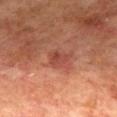Assessment: Part of a total-body skin-imaging series; this lesion was reviewed on a skin check and was not flagged for biopsy. Acquisition and patient details: Cropped from a total-body skin-imaging series; the visible field is about 15 mm. The lesion-visualizer software estimated a lesion area of about 4 mm², an outline eccentricity of about 0.65 (0 = round, 1 = elongated), and a shape-asymmetry score of about 0.35 (0 = symmetric). The lesion is on the mid back. The patient is a male aged approximately 75.